A female subject aged 73 to 77 · this image is a 15 mm lesion crop taken from a total-body photograph · located on the left lower leg.
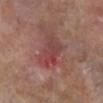– lighting: cross-polarized
– histopathology: an actinic keratosis — an indeterminate (borderline) lesion